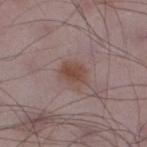No biopsy was performed on this lesion — it was imaged during a full skin examination and was not determined to be concerning.
Automated image analysis of the tile measured a lesion area of about 6.5 mm² and an outline eccentricity of about 0.65 (0 = round, 1 = elongated). The software also gave a lesion color around L≈46 a*≈18 b*≈23 in CIELAB, about 8 CIELAB-L* units darker than the surrounding skin, and a lesion-to-skin contrast of about 8 (normalized; higher = more distinct). The software also gave a classifier nevus-likeness of about 85/100 and a detector confidence of about 100 out of 100 that the crop contains a lesion.
The lesion is located on the left lower leg.
The lesion's longest dimension is about 3.5 mm.
The patient is a male roughly 50 years of age.
This is a white-light tile.
A lesion tile, about 15 mm wide, cut from a 3D total-body photograph.